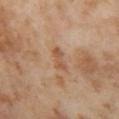Impression: Recorded during total-body skin imaging; not selected for excision or biopsy. Background: A 15 mm close-up extracted from a 3D total-body photography capture. Measured at roughly 3 mm in maximum diameter. The lesion is located on the left thigh. The subject is a female aged 53–57.